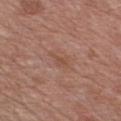No biopsy was performed on this lesion — it was imaged during a full skin examination and was not determined to be concerning.
A lesion tile, about 15 mm wide, cut from a 3D total-body photograph.
A male subject, aged approximately 65.
The lesion is located on the chest.